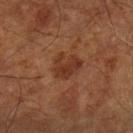follow-up: imaged on a skin check; not biopsied
subject: aged around 65
site: the left lower leg
acquisition: 15 mm crop, total-body photography
size: ≈3.5 mm
lighting: cross-polarized illumination